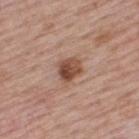biopsy status = no biopsy performed (imaged during a skin exam); patient = male, aged approximately 65; image = total-body-photography crop, ~15 mm field of view; body site = the upper back.